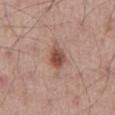  biopsy_status: not biopsied; imaged during a skin examination
  lighting: white-light
  site: front of the torso
  patient:
    sex: male
    age_approx: 65
  image:
    source: total-body photography crop
    field_of_view_mm: 15
  automated_metrics:
    area_mm2_approx: 6.0
    shape_asymmetry: 0.3
    cielab_L: 50
    cielab_a: 21
    cielab_b: 27
    vs_skin_darker_L: 12.0
    vs_skin_contrast_norm: 9.0
    nevus_likeness_0_100: 90
    lesion_detection_confidence_0_100: 100
  lesion_size:
    long_diameter_mm_approx: 3.0